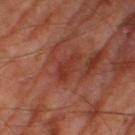Part of a total-body skin-imaging series; this lesion was reviewed on a skin check and was not flagged for biopsy. The patient is a male aged around 70. A 15 mm close-up extracted from a 3D total-body photography capture. Located on the right thigh. The total-body-photography lesion software estimated a lesion color around L≈35 a*≈28 b*≈29 in CIELAB, roughly 7 lightness units darker than nearby skin, and a lesion-to-skin contrast of about 6 (normalized; higher = more distinct). The analysis additionally found a border-irregularity index near 5.5/10, a color-variation rating of about 1.5/10, and a peripheral color-asymmetry measure near 0.5. It also reported a nevus-likeness score of about 0/100 and a lesion-detection confidence of about 70/100.